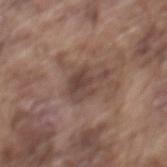Q: How large is the lesion?
A: about 4 mm
Q: What did automated image analysis measure?
A: a footprint of about 7 mm², an outline eccentricity of about 0.7 (0 = round, 1 = elongated), and two-axis asymmetry of about 0.45; an average lesion color of about L≈42 a*≈17 b*≈21 (CIELAB), a lesion–skin lightness drop of about 8, and a lesion-to-skin contrast of about 7 (normalized; higher = more distinct); a nevus-likeness score of about 5/100
Q: What kind of image is this?
A: total-body-photography crop, ~15 mm field of view
Q: Lesion location?
A: the mid back
Q: What are the patient's age and sex?
A: male, aged approximately 75